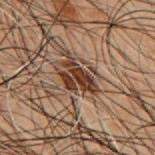Case summary:
• biopsy status · total-body-photography surveillance lesion; no biopsy
• lesion diameter · about 4.5 mm
• subject · male, approximately 50 years of age
• automated lesion analysis · a lesion color around L≈28 a*≈16 b*≈23 in CIELAB, roughly 12 lightness units darker than nearby skin, and a normalized lesion–skin contrast near 12; a border-irregularity index near 5.5/10, a color-variation rating of about 3.5/10, and radial color variation of about 1
• location · the upper back
• acquisition · ~15 mm crop, total-body skin-cancer survey
• tile lighting · cross-polarized